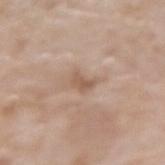No biopsy was performed on this lesion — it was imaged during a full skin examination and was not determined to be concerning. A lesion tile, about 15 mm wide, cut from a 3D total-body photograph. The lesion is located on the right forearm. A female patient approximately 75 years of age. The lesion-visualizer software estimated a border-irregularity rating of about 3.5/10, internal color variation of about 2 on a 0–10 scale, and peripheral color asymmetry of about 1. Imaged with white-light lighting. The lesion's longest dimension is about 2.5 mm.